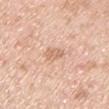No biopsy was performed on this lesion — it was imaged during a full skin examination and was not determined to be concerning.
The lesion-visualizer software estimated border irregularity of about 2.5 on a 0–10 scale and a peripheral color-asymmetry measure near 0.5.
Imaged with white-light lighting.
A 15 mm close-up extracted from a 3D total-body photography capture.
On the arm.
Longest diameter approximately 3 mm.
A male subject aged around 50.